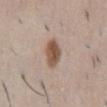Assessment:
Part of a total-body skin-imaging series; this lesion was reviewed on a skin check and was not flagged for biopsy.
Background:
On the abdomen. A male patient approximately 50 years of age. Cropped from a whole-body photographic skin survey; the tile spans about 15 mm. Automated tile analysis of the lesion measured lesion-presence confidence of about 100/100. The recorded lesion diameter is about 3.5 mm.